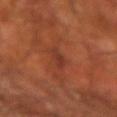Imaged during a routine full-body skin examination; the lesion was not biopsied and no histopathology is available.